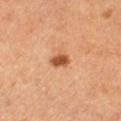An algorithmic analysis of the crop reported a lesion color around L≈53 a*≈26 b*≈38 in CIELAB and roughly 15 lightness units darker than nearby skin. Cropped from a total-body skin-imaging series; the visible field is about 15 mm. Measured at roughly 2.5 mm in maximum diameter. Imaged with cross-polarized lighting. The patient is a female about 50 years old.